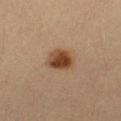{"biopsy_status": "not biopsied; imaged during a skin examination", "image": {"source": "total-body photography crop", "field_of_view_mm": 15}, "automated_metrics": {"area_mm2_approx": 8.0, "eccentricity": 0.65, "cielab_L": 40, "cielab_a": 18, "cielab_b": 30, "vs_skin_contrast_norm": 10.5, "color_variation_0_10": 5.5, "nevus_likeness_0_100": 100, "lesion_detection_confidence_0_100": 100}, "site": "left thigh", "lighting": "cross-polarized", "lesion_size": {"long_diameter_mm_approx": 3.5}, "patient": {"sex": "female", "age_approx": 30}}